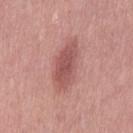The lesion was photographed on a routine skin check and not biopsied; there is no pathology result. Imaged with white-light lighting. The recorded lesion diameter is about 6.5 mm. A region of skin cropped from a whole-body photographic capture, roughly 15 mm wide. On the left thigh. The subject is a female aged approximately 40.